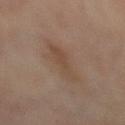Findings:
– notes — catalogued during a skin exam; not biopsied